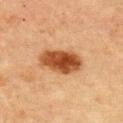anatomic site: the chest | image source: total-body-photography crop, ~15 mm field of view | automated lesion analysis: a lesion area of about 13 mm², an eccentricity of roughly 0.8, and a symmetry-axis asymmetry near 0.15; a nevus-likeness score of about 100/100 and a lesion-detection confidence of about 100/100 | lesion diameter: ~5 mm (longest diameter) | patient: male, aged approximately 65.